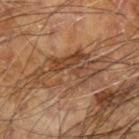notes = total-body-photography surveillance lesion; no biopsy
patient = male, in their mid-60s
site = the left forearm
lesion diameter = ~10 mm (longest diameter)
imaging modality = 15 mm crop, total-body photography